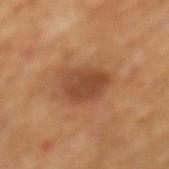workup: no biopsy performed (imaged during a skin exam) | subject: male, aged around 70 | lesion size: ≈4.5 mm | site: the mid back | image: ~15 mm tile from a whole-body skin photo | lighting: cross-polarized illumination.